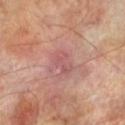Findings:
* biopsy status · imaged on a skin check; not biopsied
* acquisition · total-body-photography crop, ~15 mm field of view
* diameter · ~3 mm (longest diameter)
* image-analysis metrics · an area of roughly 4.5 mm², an outline eccentricity of about 0.8 (0 = round, 1 = elongated), and two-axis asymmetry of about 0.45; a mean CIELAB color near L≈53 a*≈25 b*≈22, roughly 6 lightness units darker than nearby skin, and a normalized border contrast of about 5; lesion-presence confidence of about 100/100
* location · the leg
* patient · male, aged approximately 70